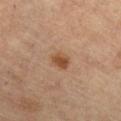Imaged during a routine full-body skin examination; the lesion was not biopsied and no histopathology is available.
The tile uses cross-polarized illumination.
The lesion's longest dimension is about 2.5 mm.
A lesion tile, about 15 mm wide, cut from a 3D total-body photograph.
The subject is a female approximately 60 years of age.
Located on the chest.